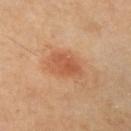Notes:
– notes · total-body-photography surveillance lesion; no biopsy
– body site · the left upper arm
– subject · male, approximately 55 years of age
– acquisition · 15 mm crop, total-body photography